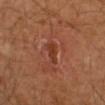Q: Was a biopsy performed?
A: no biopsy performed (imaged during a skin exam)
Q: How large is the lesion?
A: ≈2.5 mm
Q: How was this image acquired?
A: 15 mm crop, total-body photography
Q: Where on the body is the lesion?
A: the right upper arm
Q: What are the patient's age and sex?
A: male, in their mid-50s
Q: Illumination type?
A: cross-polarized illumination